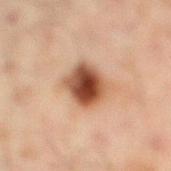Clinical impression: Part of a total-body skin-imaging series; this lesion was reviewed on a skin check and was not flagged for biopsy. Image and clinical context: A close-up tile cropped from a whole-body skin photograph, about 15 mm across. The lesion is on the leg. A male subject aged around 45. Approximately 4 mm at its widest. Captured under cross-polarized illumination.